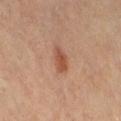This lesion was catalogued during total-body skin photography and was not selected for biopsy. Automated image analysis of the tile measured an eccentricity of roughly 0.85 and a symmetry-axis asymmetry near 0.25. The analysis additionally found about 10 CIELAB-L* units darker than the surrounding skin and a normalized lesion–skin contrast near 7.5. It also reported a border-irregularity rating of about 2.5/10 and peripheral color asymmetry of about 0.5. The analysis additionally found an automated nevus-likeness rating near 95 out of 100 and a lesion-detection confidence of about 100/100. A female patient aged 63 to 67. On the front of the torso. About 3 mm across. Imaged with cross-polarized lighting. A region of skin cropped from a whole-body photographic capture, roughly 15 mm wide.